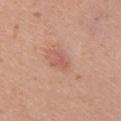{
  "biopsy_status": "not biopsied; imaged during a skin examination",
  "image": {
    "source": "total-body photography crop",
    "field_of_view_mm": 15
  },
  "patient": {
    "sex": "female",
    "age_approx": 15
  },
  "automated_metrics": {
    "vs_skin_darker_L": 7.0,
    "border_irregularity_0_10": 4.5,
    "color_variation_0_10": 0.0,
    "peripheral_color_asymmetry": 0.0
  },
  "site": "arm"
}